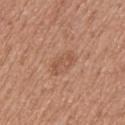Notes:
• notes · no biopsy performed (imaged during a skin exam)
• lighting · white-light
• body site · the left upper arm
• lesion size · about 3.5 mm
• image · total-body-photography crop, ~15 mm field of view
• subject · female, aged around 50